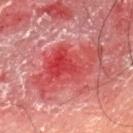anatomic site = the left thigh
lesion diameter = about 8.5 mm
subject = male, aged approximately 55
imaging modality = total-body-photography crop, ~15 mm field of view
lighting = cross-polarized illumination